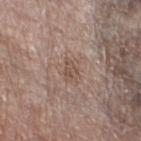Clinical impression:
Imaged during a routine full-body skin examination; the lesion was not biopsied and no histopathology is available.
Acquisition and patient details:
The lesion's longest dimension is about 2.5 mm. A female subject, approximately 75 years of age. A 15 mm close-up extracted from a 3D total-body photography capture. On the left lower leg. Automated image analysis of the tile measured a footprint of about 3 mm², an eccentricity of roughly 0.8, and a symmetry-axis asymmetry near 0.3. And it measured a normalized lesion–skin contrast near 6. And it measured an automated nevus-likeness rating near 0 out of 100 and lesion-presence confidence of about 100/100. Captured under white-light illumination.